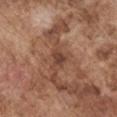Q: Patient demographics?
A: male, aged approximately 75
Q: Lesion location?
A: the left upper arm
Q: What kind of image is this?
A: ~15 mm crop, total-body skin-cancer survey